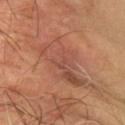No biopsy was performed on this lesion — it was imaged during a full skin examination and was not determined to be concerning. A 15 mm crop from a total-body photograph taken for skin-cancer surveillance. Captured under cross-polarized illumination. Automated tile analysis of the lesion measured an average lesion color of about L≈47 a*≈23 b*≈29 (CIELAB) and a normalized lesion–skin contrast near 6. From the left arm. A male patient, aged 58 to 62.